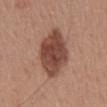Imaged during a routine full-body skin examination; the lesion was not biopsied and no histopathology is available.
This is a white-light tile.
Measured at roughly 5.5 mm in maximum diameter.
A male subject, aged approximately 55.
The lesion is located on the mid back.
A 15 mm crop from a total-body photograph taken for skin-cancer surveillance.
The lesion-visualizer software estimated a footprint of about 20 mm² and a shape-asymmetry score of about 0.1 (0 = symmetric). The analysis additionally found an average lesion color of about L≈45 a*≈22 b*≈26 (CIELAB), about 14 CIELAB-L* units darker than the surrounding skin, and a normalized border contrast of about 10.5. The software also gave a border-irregularity rating of about 1.5/10.